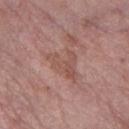Recorded during total-body skin imaging; not selected for excision or biopsy. From the left thigh. Longest diameter approximately 4.5 mm. A female subject, approximately 85 years of age or older. Cropped from a total-body skin-imaging series; the visible field is about 15 mm. This is a white-light tile.